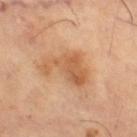- follow-up: catalogued during a skin exam; not biopsied
- lesion diameter: ~6 mm (longest diameter)
- imaging modality: total-body-photography crop, ~15 mm field of view
- patient: male, approximately 65 years of age
- automated lesion analysis: a color-variation rating of about 4/10 and peripheral color asymmetry of about 1
- lighting: cross-polarized
- anatomic site: the right thigh